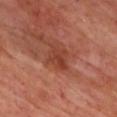follow-up: catalogued during a skin exam; not biopsied | body site: the chest | lesion size: ~3 mm (longest diameter) | subject: male, aged approximately 65 | illumination: cross-polarized illumination | image: ~15 mm crop, total-body skin-cancer survey | automated metrics: a lesion area of about 6 mm² and an outline eccentricity of about 0.55 (0 = round, 1 = elongated); a mean CIELAB color near L≈39 a*≈27 b*≈30.